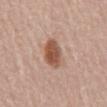Clinical impression:
The lesion was tiled from a total-body skin photograph and was not biopsied.
Acquisition and patient details:
Imaged with white-light lighting. Automated tile analysis of the lesion measured an area of roughly 9 mm² and a shape-asymmetry score of about 0.15 (0 = symmetric). And it measured a border-irregularity rating of about 2/10, a color-variation rating of about 4/10, and a peripheral color-asymmetry measure near 1. Cropped from a total-body skin-imaging series; the visible field is about 15 mm. About 5 mm across. On the mid back. The patient is a male about 60 years old.